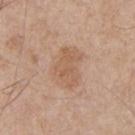Q: What kind of image is this?
A: ~15 mm crop, total-body skin-cancer survey
Q: How was the tile lit?
A: white-light
Q: Patient demographics?
A: male, approximately 55 years of age
Q: What is the anatomic site?
A: the upper back